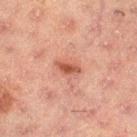Part of a total-body skin-imaging series; this lesion was reviewed on a skin check and was not flagged for biopsy. A lesion tile, about 15 mm wide, cut from a 3D total-body photograph. The lesion is on the right thigh. A male subject about 50 years old.